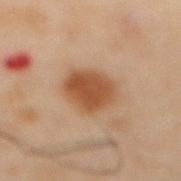{
  "biopsy_status": "not biopsied; imaged during a skin examination",
  "site": "abdomen",
  "lesion_size": {
    "long_diameter_mm_approx": 4.5
  },
  "image": {
    "source": "total-body photography crop",
    "field_of_view_mm": 15
  },
  "lighting": "cross-polarized",
  "patient": {
    "sex": "male",
    "age_approx": 50
  }
}